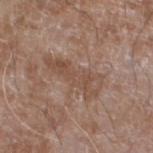• biopsy status — no biopsy performed (imaged during a skin exam)
• diameter — ≈6.5 mm
• lighting — white-light illumination
• subject — male, approximately 60 years of age
• body site — the left lower leg
• image source — 15 mm crop, total-body photography